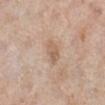Findings:
• biopsy status · total-body-photography surveillance lesion; no biopsy
• acquisition · ~15 mm tile from a whole-body skin photo
• site · the right lower leg
• patient · female, roughly 65 years of age
• size · ≈3.5 mm